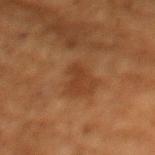Automated tile analysis of the lesion measured a footprint of about 2.5 mm², a shape eccentricity near 0.9, and a shape-asymmetry score of about 0.35 (0 = symmetric). It also reported a lesion–skin lightness drop of about 5 and a normalized lesion–skin contrast near 5.5.
Longest diameter approximately 2.5 mm.
The lesion is on the chest.
A 15 mm close-up extracted from a 3D total-body photography capture.
A female patient approximately 80 years of age.